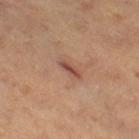workup: no biopsy performed (imaged during a skin exam) | lesion size: ≈3 mm | acquisition: total-body-photography crop, ~15 mm field of view | patient: female, aged approximately 50 | location: the right thigh | illumination: cross-polarized | image-analysis metrics: a footprint of about 2 mm², a shape eccentricity near 0.95, and a shape-asymmetry score of about 0.3 (0 = symmetric); an average lesion color of about L≈46 a*≈24 b*≈25 (CIELAB), a lesion–skin lightness drop of about 11, and a normalized border contrast of about 9.